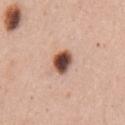Q: Was a biopsy performed?
A: imaged on a skin check; not biopsied
Q: Illumination type?
A: white-light
Q: Lesion location?
A: the left upper arm
Q: How was this image acquired?
A: 15 mm crop, total-body photography
Q: How large is the lesion?
A: about 3 mm
Q: What did automated image analysis measure?
A: a footprint of about 6 mm² and an outline eccentricity of about 0.5 (0 = round, 1 = elongated); an automated nevus-likeness rating near 100 out of 100
Q: Patient demographics?
A: female, aged 43–47